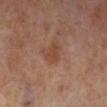Clinical impression:
The lesion was tiled from a total-body skin photograph and was not biopsied.
Background:
The recorded lesion diameter is about 3 mm. Cropped from a whole-body photographic skin survey; the tile spans about 15 mm. The lesion is located on the right lower leg. A female subject, aged 53–57. The tile uses cross-polarized illumination.